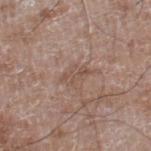Assessment:
The lesion was tiled from a total-body skin photograph and was not biopsied.
Image and clinical context:
Imaged with white-light lighting. The lesion's longest dimension is about 3 mm. The subject is a male aged 58–62. The lesion-visualizer software estimated a lesion color around L≈50 a*≈18 b*≈25 in CIELAB, about 7 CIELAB-L* units darker than the surrounding skin, and a normalized lesion–skin contrast near 5.5. The analysis additionally found a classifier nevus-likeness of about 0/100 and lesion-presence confidence of about 95/100. On the right lower leg. This image is a 15 mm lesion crop taken from a total-body photograph.